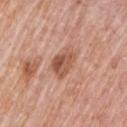Clinical impression: This lesion was catalogued during total-body skin photography and was not selected for biopsy. Image and clinical context: On the left upper arm. A 15 mm close-up extracted from a 3D total-body photography capture. The recorded lesion diameter is about 3.5 mm. A male patient in their mid-70s. This is a white-light tile.